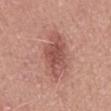| feature | finding |
|---|---|
| workup | total-body-photography surveillance lesion; no biopsy |
| patient | male, aged 48–52 |
| illumination | white-light illumination |
| diameter | ~7.5 mm (longest diameter) |
| image source | 15 mm crop, total-body photography |
| location | the back |